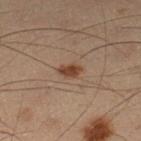A male subject aged 53 to 57.
From the left lower leg.
Captured under cross-polarized illumination.
A region of skin cropped from a whole-body photographic capture, roughly 15 mm wide.
The lesion-visualizer software estimated a footprint of about 3.5 mm² and two-axis asymmetry of about 0.3. And it measured a lesion color around L≈34 a*≈16 b*≈24 in CIELAB, a lesion–skin lightness drop of about 9, and a lesion-to-skin contrast of about 9 (normalized; higher = more distinct).
The lesion's longest dimension is about 2.5 mm.